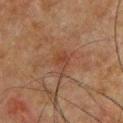workup: no biopsy performed (imaged during a skin exam)